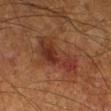{"biopsy_status": "not biopsied; imaged during a skin examination", "automated_metrics": {"area_mm2_approx": 18.0, "eccentricity": 0.85, "shape_asymmetry": 0.4, "border_irregularity_0_10": 5.0, "color_variation_0_10": 6.5, "peripheral_color_asymmetry": 2.0}, "image": {"source": "total-body photography crop", "field_of_view_mm": 15}, "site": "leg", "lighting": "cross-polarized", "patient": {"sex": "male", "age_approx": 60}}